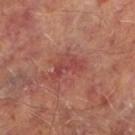Part of a total-body skin-imaging series; this lesion was reviewed on a skin check and was not flagged for biopsy. Cropped from a whole-body photographic skin survey; the tile spans about 15 mm. Automated image analysis of the tile measured a mean CIELAB color near L≈44 a*≈29 b*≈25, a lesion–skin lightness drop of about 7, and a normalized lesion–skin contrast near 6. And it measured internal color variation of about 3 on a 0–10 scale and a peripheral color-asymmetry measure near 1. The analysis additionally found an automated nevus-likeness rating near 0 out of 100 and a lesion-detection confidence of about 100/100. On the left lower leg. The tile uses cross-polarized illumination. A male subject aged 63–67.